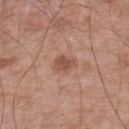workup=no biopsy performed (imaged during a skin exam); image=~15 mm crop, total-body skin-cancer survey; anatomic site=the upper back; lesion diameter=~2.5 mm (longest diameter); tile lighting=white-light; TBP lesion metrics=a border-irregularity rating of about 2/10, internal color variation of about 3 on a 0–10 scale, and peripheral color asymmetry of about 1; subject=male, in their mid- to late 60s.